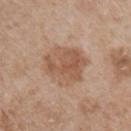This lesion was catalogued during total-body skin photography and was not selected for biopsy. The lesion is located on the chest. The subject is a male aged around 65. Cropped from a whole-body photographic skin survey; the tile spans about 15 mm. The lesion's longest dimension is about 5.5 mm. This is a white-light tile. Automated image analysis of the tile measured an average lesion color of about L≈56 a*≈19 b*≈30 (CIELAB), a lesion–skin lightness drop of about 9, and a lesion-to-skin contrast of about 6.5 (normalized; higher = more distinct). It also reported a border-irregularity index near 2/10 and radial color variation of about 1.5.